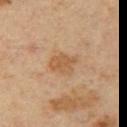Findings:
- follow-up: no biopsy performed (imaged during a skin exam)